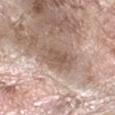follow-up: no biopsy performed (imaged during a skin exam) | imaging modality: total-body-photography crop, ~15 mm field of view | body site: the arm | patient: female, about 75 years old | diameter: ~4.5 mm (longest diameter) | lighting: white-light illumination.